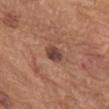The lesion was tiled from a total-body skin photograph and was not biopsied.
This is a white-light tile.
The recorded lesion diameter is about 3 mm.
The subject is a female aged 63 to 67.
Automated image analysis of the tile measured an area of roughly 4.5 mm² and a shape-asymmetry score of about 0.15 (0 = symmetric). It also reported a mean CIELAB color near L≈43 a*≈21 b*≈25, roughly 12 lightness units darker than nearby skin, and a normalized border contrast of about 9.5. It also reported a border-irregularity rating of about 1.5/10, a color-variation rating of about 3/10, and peripheral color asymmetry of about 1. The analysis additionally found a classifier nevus-likeness of about 70/100 and a lesion-detection confidence of about 100/100.
A 15 mm crop from a total-body photograph taken for skin-cancer surveillance.
The lesion is located on the abdomen.